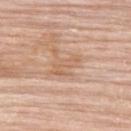follow-up: total-body-photography surveillance lesion; no biopsy | patient: female, roughly 70 years of age | tile lighting: white-light | image: ~15 mm crop, total-body skin-cancer survey | automated metrics: an eccentricity of roughly 0.9; a normalized border contrast of about 5; a border-irregularity index near 6.5/10, a within-lesion color-variation index near 0/10, and peripheral color asymmetry of about 0; a nevus-likeness score of about 0/100 and lesion-presence confidence of about 75/100 | body site: the upper back | lesion size: ~3.5 mm (longest diameter).